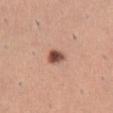Q: Was this lesion biopsied?
A: total-body-photography surveillance lesion; no biopsy
Q: Illumination type?
A: white-light
Q: What kind of image is this?
A: ~15 mm crop, total-body skin-cancer survey
Q: Who is the patient?
A: female, aged approximately 30
Q: Where on the body is the lesion?
A: the leg
Q: What did automated image analysis measure?
A: an average lesion color of about L≈51 a*≈22 b*≈28 (CIELAB), about 17 CIELAB-L* units darker than the surrounding skin, and a normalized lesion–skin contrast near 11; a border-irregularity rating of about 2/10 and a within-lesion color-variation index near 5.5/10; a detector confidence of about 100 out of 100 that the crop contains a lesion